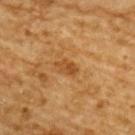The lesion was tiled from a total-body skin photograph and was not biopsied.
Cropped from a total-body skin-imaging series; the visible field is about 15 mm.
Imaged with cross-polarized lighting.
An algorithmic analysis of the crop reported a shape eccentricity near 0.85 and two-axis asymmetry of about 0.25. The software also gave about 8 CIELAB-L* units darker than the surrounding skin and a normalized border contrast of about 6.5.
Longest diameter approximately 3 mm.
The lesion is located on the back.
A male patient about 85 years old or older.